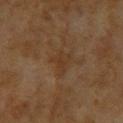follow-up = imaged on a skin check; not biopsied
subject = female, roughly 60 years of age
acquisition = 15 mm crop, total-body photography
lesion diameter = ≈3 mm
body site = the upper back
illumination = cross-polarized